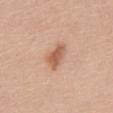follow-up = catalogued during a skin exam; not biopsied
location = the mid back
imaging modality = 15 mm crop, total-body photography
subject = female, about 65 years old